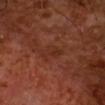* notes · catalogued during a skin exam; not biopsied
* image · ~15 mm crop, total-body skin-cancer survey
* patient · male, aged 63–67